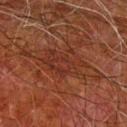biopsy status = catalogued during a skin exam; not biopsied
tile lighting = cross-polarized
image-analysis metrics = border irregularity of about 6 on a 0–10 scale, a within-lesion color-variation index near 3/10, and peripheral color asymmetry of about 1
lesion size = ~10 mm (longest diameter)
patient = male, approximately 80 years of age
site = the arm
acquisition = total-body-photography crop, ~15 mm field of view